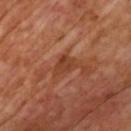imaging modality — ~15 mm tile from a whole-body skin photo | subject — male, aged 63–67 | TBP lesion metrics — border irregularity of about 3.5 on a 0–10 scale; a detector confidence of about 100 out of 100 that the crop contains a lesion | lesion size — about 3 mm.